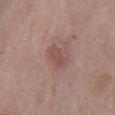The lesion was photographed on a routine skin check and not biopsied; there is no pathology result. Cropped from a whole-body photographic skin survey; the tile spans about 15 mm. The patient is a male approximately 75 years of age. The lesion is on the mid back. Imaged with white-light lighting. The recorded lesion diameter is about 2.5 mm.